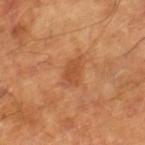The lesion was photographed on a routine skin check and not biopsied; there is no pathology result. This is a cross-polarized tile. Approximately 3.5 mm at its widest. A 15 mm close-up extracted from a 3D total-body photography capture. A male subject approximately 65 years of age.